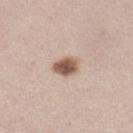This lesion was catalogued during total-body skin photography and was not selected for biopsy.